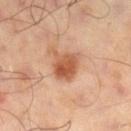| feature | finding |
|---|---|
| follow-up | no biopsy performed (imaged during a skin exam) |
| image | ~15 mm tile from a whole-body skin photo |
| body site | the right lower leg |
| patient | male, about 65 years old |
| lighting | cross-polarized |
| size | ~3.5 mm (longest diameter) |